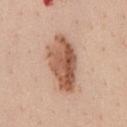Q: How was the tile lit?
A: white-light illumination
Q: Lesion location?
A: the chest
Q: What are the patient's age and sex?
A: male, aged approximately 40
Q: Automated lesion metrics?
A: a footprint of about 20 mm², an eccentricity of roughly 0.9, and a symmetry-axis asymmetry near 0.2; a border-irregularity rating of about 2.5/10, a color-variation rating of about 7/10, and radial color variation of about 3
Q: What is the lesion's diameter?
A: about 7.5 mm
Q: How was this image acquired?
A: 15 mm crop, total-body photography